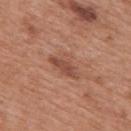The lesion was tiled from a total-body skin photograph and was not biopsied. A male patient, roughly 70 years of age. The lesion is located on the mid back. Cropped from a total-body skin-imaging series; the visible field is about 15 mm. Longest diameter approximately 4.5 mm. Captured under white-light illumination.